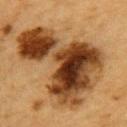Clinical impression:
Recorded during total-body skin imaging; not selected for excision or biopsy.
Background:
Measured at roughly 11.5 mm in maximum diameter. Captured under cross-polarized illumination. A male subject aged 83 or over. A lesion tile, about 15 mm wide, cut from a 3D total-body photograph. The lesion is on the left upper arm.